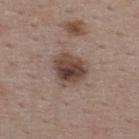Imaged during a routine full-body skin examination; the lesion was not biopsied and no histopathology is available.
A 15 mm crop from a total-body photograph taken for skin-cancer surveillance.
A female patient about 45 years old.
On the upper back.
The lesion's longest dimension is about 3.5 mm.
Captured under white-light illumination.